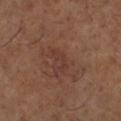The lesion was tiled from a total-body skin photograph and was not biopsied. Located on the leg. A 15 mm close-up extracted from a 3D total-body photography capture. The patient is a male about 65 years old. Longest diameter approximately 3 mm. The tile uses cross-polarized illumination.